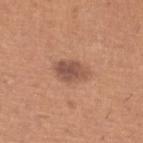The recorded lesion diameter is about 4 mm. This image is a 15 mm lesion crop taken from a total-body photograph. This is a white-light tile. The lesion is on the leg. A male patient roughly 40 years of age.